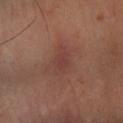| feature | finding |
|---|---|
| biopsy status | no biopsy performed (imaged during a skin exam) |
| location | the arm |
| lesion diameter | ≈3.5 mm |
| subject | aged 63–67 |
| image source | 15 mm crop, total-body photography |
| TBP lesion metrics | a lesion area of about 5.5 mm², an eccentricity of roughly 0.85, and two-axis asymmetry of about 0.3; a nevus-likeness score of about 0/100 |
| tile lighting | cross-polarized illumination |